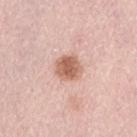Impression:
Imaged during a routine full-body skin examination; the lesion was not biopsied and no histopathology is available.
Clinical summary:
A roughly 15 mm field-of-view crop from a total-body skin photograph. About 3 mm across. Automated tile analysis of the lesion measured an outline eccentricity of about 0.45 (0 = round, 1 = elongated) and a shape-asymmetry score of about 0.2 (0 = symmetric). This is a white-light tile. A female subject in their mid-60s. The lesion is on the lower back.